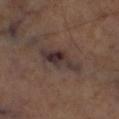follow-up — no biopsy performed (imaged during a skin exam)
site — the leg
lesion diameter — about 6 mm
lighting — cross-polarized
automated lesion analysis — an area of roughly 10 mm², a shape eccentricity near 0.9, and a shape-asymmetry score of about 0.3 (0 = symmetric); an average lesion color of about L≈32 a*≈14 b*≈16 (CIELAB), about 9 CIELAB-L* units darker than the surrounding skin, and a normalized border contrast of about 10.5; a nevus-likeness score of about 5/100
imaging modality — total-body-photography crop, ~15 mm field of view